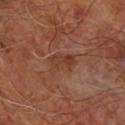Captured under cross-polarized illumination.
A close-up tile cropped from a whole-body skin photograph, about 15 mm across.
From the right forearm.
A male patient aged 68–72.
The lesion-visualizer software estimated a lesion area of about 6.5 mm², an eccentricity of roughly 0.75, and a symmetry-axis asymmetry near 0.35. The analysis additionally found an average lesion color of about L≈37 a*≈22 b*≈28 (CIELAB), roughly 6 lightness units darker than nearby skin, and a normalized border contrast of about 6. It also reported a border-irregularity index near 4/10 and a within-lesion color-variation index near 3/10. The analysis additionally found a classifier nevus-likeness of about 0/100 and a detector confidence of about 100 out of 100 that the crop contains a lesion.
Approximately 3.5 mm at its widest.